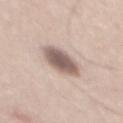{"biopsy_status": "not biopsied; imaged during a skin examination", "lesion_size": {"long_diameter_mm_approx": 5.0}, "automated_metrics": {"area_mm2_approx": 11.0, "eccentricity": 0.75, "nevus_likeness_0_100": 45}, "site": "mid back", "lighting": "white-light", "patient": {"sex": "male", "age_approx": 45}, "image": {"source": "total-body photography crop", "field_of_view_mm": 15}}